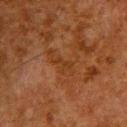| key | value |
|---|---|
| anatomic site | the upper back |
| imaging modality | 15 mm crop, total-body photography |
| size | about 2.5 mm |
| patient | male, roughly 60 years of age |
| lighting | cross-polarized |
| image-analysis metrics | an area of roughly 2 mm², a shape eccentricity near 0.95, and two-axis asymmetry of about 0.55; a border-irregularity index near 6/10; a nevus-likeness score of about 0/100 |